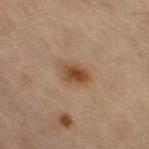  biopsy_status: not biopsied; imaged during a skin examination
  image:
    source: total-body photography crop
    field_of_view_mm: 15
  site: leg
  patient:
    sex: female
    age_approx: 65